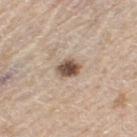Part of a total-body skin-imaging series; this lesion was reviewed on a skin check and was not flagged for biopsy. An algorithmic analysis of the crop reported a classifier nevus-likeness of about 100/100 and a lesion-detection confidence of about 100/100. The lesion's longest dimension is about 3 mm. A female subject aged 68–72. On the left thigh. Imaged with white-light lighting. A 15 mm close-up extracted from a 3D total-body photography capture.